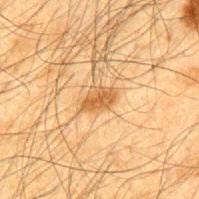The lesion was tiled from a total-body skin photograph and was not biopsied.
This image is a 15 mm lesion crop taken from a total-body photograph.
Measured at roughly 3 mm in maximum diameter.
A male subject aged 63–67.
Imaged with cross-polarized lighting.
The lesion is on the mid back.